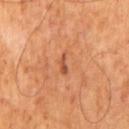<case>
  <biopsy_status>not biopsied; imaged during a skin examination</biopsy_status>
</case>